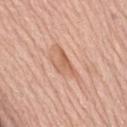This lesion was catalogued during total-body skin photography and was not selected for biopsy. Located on the abdomen. Cropped from a total-body skin-imaging series; the visible field is about 15 mm. Captured under white-light illumination. A female subject, roughly 75 years of age.